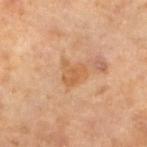Impression: The lesion was photographed on a routine skin check and not biopsied; there is no pathology result. Context: The lesion is on the left lower leg. A female patient aged 68 to 72. This image is a 15 mm lesion crop taken from a total-body photograph.